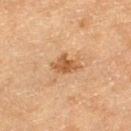Assessment:
Captured during whole-body skin photography for melanoma surveillance; the lesion was not biopsied.
Image and clinical context:
Approximately 3.5 mm at its widest. Automated image analysis of the tile measured a symmetry-axis asymmetry near 0.3. The analysis additionally found internal color variation of about 3 on a 0–10 scale and peripheral color asymmetry of about 1. A female subject, about 55 years old. Captured under cross-polarized illumination. The lesion is located on the left thigh. This image is a 15 mm lesion crop taken from a total-body photograph.